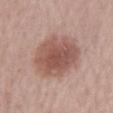Findings:
– patient: male, in their mid- to late 60s
– body site: the lower back
– image source: ~15 mm tile from a whole-body skin photo